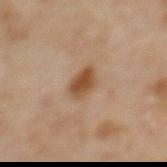| key | value |
|---|---|
| follow-up | no biopsy performed (imaged during a skin exam) |
| acquisition | 15 mm crop, total-body photography |
| body site | the upper back |
| patient | female, aged around 60 |